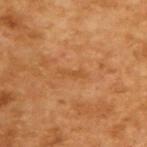Clinical impression:
Captured during whole-body skin photography for melanoma surveillance; the lesion was not biopsied.
Clinical summary:
Imaged with cross-polarized lighting. A 15 mm crop from a total-body photograph taken for skin-cancer surveillance. An algorithmic analysis of the crop reported an outline eccentricity of about 0.95 (0 = round, 1 = elongated) and two-axis asymmetry of about 0.3. The analysis additionally found a mean CIELAB color near L≈51 a*≈24 b*≈41, a lesion–skin lightness drop of about 6, and a normalized lesion–skin contrast near 4.5. The software also gave a nevus-likeness score of about 0/100. A male subject aged 63 to 67. The recorded lesion diameter is about 3 mm.